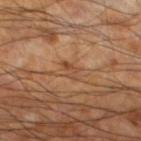Imaged during a routine full-body skin examination; the lesion was not biopsied and no histopathology is available.
Captured under cross-polarized illumination.
Automated image analysis of the tile measured roughly 7 lightness units darker than nearby skin and a normalized border contrast of about 5.5. It also reported border irregularity of about 6.5 on a 0–10 scale, a within-lesion color-variation index near 0/10, and radial color variation of about 0. And it measured an automated nevus-likeness rating near 0 out of 100 and a detector confidence of about 90 out of 100 that the crop contains a lesion.
A male patient, aged approximately 60.
On the left lower leg.
The recorded lesion diameter is about 2.5 mm.
A roughly 15 mm field-of-view crop from a total-body skin photograph.